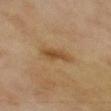{
  "biopsy_status": "not biopsied; imaged during a skin examination",
  "image": {
    "source": "total-body photography crop",
    "field_of_view_mm": 15
  },
  "site": "left thigh",
  "lighting": "cross-polarized",
  "lesion_size": {
    "long_diameter_mm_approx": 4.0
  },
  "automated_metrics": {
    "area_mm2_approx": 5.0,
    "eccentricity": 0.95,
    "shape_asymmetry": 0.25,
    "border_irregularity_0_10": 3.0,
    "color_variation_0_10": 1.5,
    "peripheral_color_asymmetry": 0.5,
    "lesion_detection_confidence_0_100": 100
  },
  "patient": {
    "sex": "female",
    "age_approx": 60
  }
}